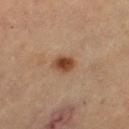Recorded during total-body skin imaging; not selected for excision or biopsy.
A female subject approximately 70 years of age.
This is a cross-polarized tile.
The lesion is on the left thigh.
Longest diameter approximately 3 mm.
A 15 mm close-up tile from a total-body photography series done for melanoma screening.